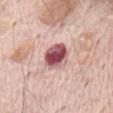biopsy status = total-body-photography surveillance lesion; no biopsy
image = ~15 mm crop, total-body skin-cancer survey
patient = male, aged 68 to 72
tile lighting = white-light illumination
size = ~4 mm (longest diameter)
site = the chest
TBP lesion metrics = a mean CIELAB color near L≈54 a*≈28 b*≈19 and a normalized border contrast of about 13; a nevus-likeness score of about 40/100 and a detector confidence of about 100 out of 100 that the crop contains a lesion